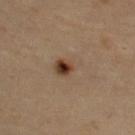Captured during whole-body skin photography for melanoma surveillance; the lesion was not biopsied. A 15 mm close-up tile from a total-body photography series done for melanoma screening. The lesion is located on the upper back. A male patient, aged 33–37.